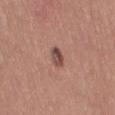No biopsy was performed on this lesion — it was imaged during a full skin examination and was not determined to be concerning. The lesion's longest dimension is about 2.5 mm. The lesion is located on the leg. A close-up tile cropped from a whole-body skin photograph, about 15 mm across. The patient is a female roughly 30 years of age. The total-body-photography lesion software estimated an area of roughly 3.5 mm², a shape eccentricity near 0.85, and a symmetry-axis asymmetry near 0.3. The software also gave a mean CIELAB color near L≈47 a*≈21 b*≈24 and a normalized lesion–skin contrast near 9. The software also gave border irregularity of about 2.5 on a 0–10 scale, internal color variation of about 5 on a 0–10 scale, and peripheral color asymmetry of about 2. The analysis additionally found a detector confidence of about 100 out of 100 that the crop contains a lesion.